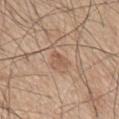The lesion was tiled from a total-body skin photograph and was not biopsied. The lesion is located on the back. The lesion's longest dimension is about 3 mm. A 15 mm crop from a total-body photograph taken for skin-cancer surveillance. This is a white-light tile. A male patient in their mid-60s. The lesion-visualizer software estimated a lesion area of about 3 mm², an outline eccentricity of about 0.9 (0 = round, 1 = elongated), and a shape-asymmetry score of about 0.35 (0 = symmetric). The software also gave an average lesion color of about L≈56 a*≈19 b*≈30 (CIELAB). The software also gave a within-lesion color-variation index near 1/10 and radial color variation of about 0.5.